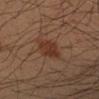Recorded during total-body skin imaging; not selected for excision or biopsy. A lesion tile, about 15 mm wide, cut from a 3D total-body photograph. Automated tile analysis of the lesion measured border irregularity of about 2.5 on a 0–10 scale, internal color variation of about 2 on a 0–10 scale, and a peripheral color-asymmetry measure near 0.5. Approximately 3 mm at its widest. On the left forearm. The subject is a male in their mid-30s. The tile uses cross-polarized illumination.